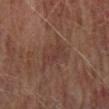{
  "biopsy_status": "not biopsied; imaged during a skin examination",
  "site": "back",
  "image": {
    "source": "total-body photography crop",
    "field_of_view_mm": 15
  },
  "patient": {
    "sex": "male",
    "age_approx": 75
  }
}